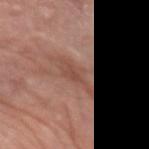Recorded during total-body skin imaging; not selected for excision or biopsy.
A male subject, approximately 50 years of age.
The lesion is on the arm.
This image is a 15 mm lesion crop taken from a total-body photograph.
The lesion's longest dimension is about 5 mm.